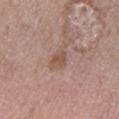No biopsy was performed on this lesion — it was imaged during a full skin examination and was not determined to be concerning.
The subject is a female in their mid-60s.
Approximately 2.5 mm at its widest.
From the left lower leg.
The tile uses white-light illumination.
This image is a 15 mm lesion crop taken from a total-body photograph.
Automated tile analysis of the lesion measured an area of roughly 3.5 mm², an eccentricity of roughly 0.75, and a shape-asymmetry score of about 0.3 (0 = symmetric). The software also gave border irregularity of about 3 on a 0–10 scale, internal color variation of about 1 on a 0–10 scale, and peripheral color asymmetry of about 0.5.